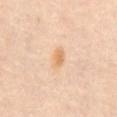Recorded during total-body skin imaging; not selected for excision or biopsy. Located on the chest. A 15 mm crop from a total-body photograph taken for skin-cancer surveillance. A female patient aged approximately 60. Measured at roughly 3 mm in maximum diameter. Imaged with cross-polarized lighting.